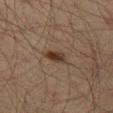Case summary:
* follow-up: imaged on a skin check; not biopsied
* lesion size: about 3 mm
* tile lighting: cross-polarized
* image source: 15 mm crop, total-body photography
* anatomic site: the leg
* subject: male, aged around 45